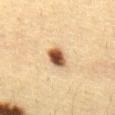{
  "biopsy_status": "not biopsied; imaged during a skin examination",
  "patient": {
    "sex": "female",
    "age_approx": 30
  },
  "image": {
    "source": "total-body photography crop",
    "field_of_view_mm": 15
  },
  "automated_metrics": {
    "area_mm2_approx": 6.0,
    "eccentricity": 0.65,
    "shape_asymmetry": 0.15,
    "cielab_L": 51,
    "cielab_a": 19,
    "cielab_b": 33,
    "vs_skin_darker_L": 20.0,
    "lesion_detection_confidence_0_100": 100
  },
  "site": "abdomen"
}